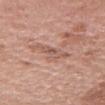Captured during whole-body skin photography for melanoma surveillance; the lesion was not biopsied.
An algorithmic analysis of the crop reported a lesion area of about 4.5 mm² and an eccentricity of roughly 0.95. And it measured a color-variation rating of about 2/10 and peripheral color asymmetry of about 0.5. The analysis additionally found an automated nevus-likeness rating near 0 out of 100 and lesion-presence confidence of about 55/100.
A female subject approximately 65 years of age.
This is a white-light tile.
The lesion's longest dimension is about 4.5 mm.
A 15 mm crop from a total-body photograph taken for skin-cancer surveillance.
The lesion is located on the head or neck.